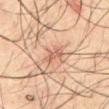Clinical impression: The lesion was photographed on a routine skin check and not biopsied; there is no pathology result. Context: A male subject, aged around 60. A lesion tile, about 15 mm wide, cut from a 3D total-body photograph. Located on the mid back.